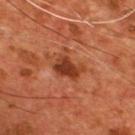biopsy_status: not biopsied; imaged during a skin examination
lighting: cross-polarized
patient:
  sex: male
  age_approx: 55
site: chest
image:
  source: total-body photography crop
  field_of_view_mm: 15
lesion_size:
  long_diameter_mm_approx: 3.5